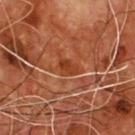On the chest. A roughly 15 mm field-of-view crop from a total-body skin photograph. Approximately 3 mm at its widest. Imaged with cross-polarized lighting. Automated image analysis of the tile measured a mean CIELAB color near L≈38 a*≈28 b*≈35, a lesion–skin lightness drop of about 7, and a normalized border contrast of about 6.5. The analysis additionally found peripheral color asymmetry of about 1. And it measured a nevus-likeness score of about 0/100 and a lesion-detection confidence of about 95/100. A male subject, in their mid- to late 50s.